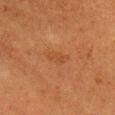  image:
    source: total-body photography crop
    field_of_view_mm: 15
  site: head or neck
  patient:
    sex: female
    age_approx: 50